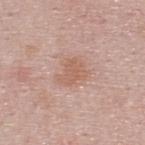Clinical impression: Imaged during a routine full-body skin examination; the lesion was not biopsied and no histopathology is available. Clinical summary: Cropped from a whole-body photographic skin survey; the tile spans about 15 mm. The lesion is located on the upper back. A male patient about 25 years old.